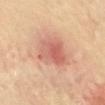Q: Was a biopsy performed?
A: imaged on a skin check; not biopsied
Q: How large is the lesion?
A: ~4.5 mm (longest diameter)
Q: Who is the patient?
A: female, about 60 years old
Q: What is the imaging modality?
A: ~15 mm tile from a whole-body skin photo
Q: What did automated image analysis measure?
A: a lesion color around L≈61 a*≈26 b*≈30 in CIELAB and roughly 10 lightness units darker than nearby skin; border irregularity of about 2 on a 0–10 scale, a color-variation rating of about 6/10, and peripheral color asymmetry of about 2
Q: Where on the body is the lesion?
A: the abdomen
Q: What lighting was used for the tile?
A: cross-polarized